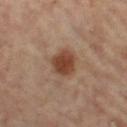Notes:
* follow-up: no biopsy performed (imaged during a skin exam)
* location: the right leg
* image source: total-body-photography crop, ~15 mm field of view
* patient: female, in their mid-60s
* size: ≈3.5 mm
* tile lighting: cross-polarized